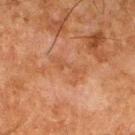Q: Was this lesion biopsied?
A: catalogued during a skin exam; not biopsied
Q: Automated lesion metrics?
A: a border-irregularity index near 7.5/10 and a color-variation rating of about 0.5/10; an automated nevus-likeness rating near 0 out of 100 and a lesion-detection confidence of about 100/100
Q: What kind of image is this?
A: total-body-photography crop, ~15 mm field of view
Q: What is the lesion's diameter?
A: ≈4.5 mm
Q: What is the anatomic site?
A: the right thigh
Q: Who is the patient?
A: male, aged 78 to 82